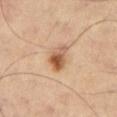biopsy status: imaged on a skin check; not biopsied
image: ~15 mm tile from a whole-body skin photo
lighting: cross-polarized illumination
anatomic site: the right thigh
image-analysis metrics: roughly 14 lightness units darker than nearby skin and a lesion-to-skin contrast of about 9 (normalized; higher = more distinct); a border-irregularity rating of about 3/10 and internal color variation of about 9.5 on a 0–10 scale; a classifier nevus-likeness of about 95/100 and a detector confidence of about 100 out of 100 that the crop contains a lesion